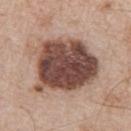notes — no biopsy performed (imaged during a skin exam); subject — male, about 65 years old; lesion size — about 7.5 mm; tile lighting — white-light illumination; location — the right upper arm; image — ~15 mm tile from a whole-body skin photo.